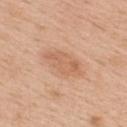Assessment:
Part of a total-body skin-imaging series; this lesion was reviewed on a skin check and was not flagged for biopsy.
Acquisition and patient details:
Automated tile analysis of the lesion measured an automated nevus-likeness rating near 30 out of 100. A 15 mm close-up extracted from a 3D total-body photography capture. A female patient, approximately 40 years of age. The tile uses white-light illumination. About 5 mm across. On the upper back.